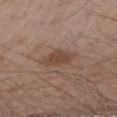• biopsy status — catalogued during a skin exam; not biopsied
• location — the right forearm
• acquisition — total-body-photography crop, ~15 mm field of view
• patient — male, aged around 55
• illumination — white-light illumination
• image-analysis metrics — border irregularity of about 2 on a 0–10 scale and a within-lesion color-variation index near 2/10; a classifier nevus-likeness of about 65/100 and a lesion-detection confidence of about 100/100
• lesion diameter — about 3 mm